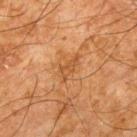Notes:
* image source: 15 mm crop, total-body photography
* illumination: cross-polarized
* location: the upper back
* subject: male, aged approximately 65
* automated metrics: roughly 7 lightness units darker than nearby skin and a normalized border contrast of about 5.5